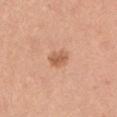Impression:
Captured during whole-body skin photography for melanoma surveillance; the lesion was not biopsied.
Image and clinical context:
A 15 mm crop from a total-body photograph taken for skin-cancer surveillance. The patient is a male aged 28–32. The tile uses white-light illumination. The lesion is located on the left upper arm.